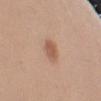This lesion was catalogued during total-body skin photography and was not selected for biopsy.
A 15 mm close-up tile from a total-body photography series done for melanoma screening.
Captured under white-light illumination.
Automated image analysis of the tile measured a lesion area of about 4.5 mm², an eccentricity of roughly 0.6, and a shape-asymmetry score of about 0.15 (0 = symmetric). The analysis additionally found an average lesion color of about L≈56 a*≈21 b*≈31 (CIELAB), roughly 10 lightness units darker than nearby skin, and a lesion-to-skin contrast of about 7 (normalized; higher = more distinct).
The patient is a male aged around 45.
Located on the arm.